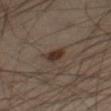No biopsy was performed on this lesion — it was imaged during a full skin examination and was not determined to be concerning. Located on the right thigh. A male patient, aged 53–57. A 15 mm crop from a total-body photograph taken for skin-cancer surveillance. Measured at roughly 2.5 mm in maximum diameter. An algorithmic analysis of the crop reported internal color variation of about 1.5 on a 0–10 scale and radial color variation of about 0.5.